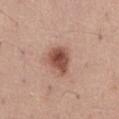Recorded during total-body skin imaging; not selected for excision or biopsy. The lesion is on the front of the torso. Cropped from a total-body skin-imaging series; the visible field is about 15 mm. Imaged with white-light lighting. The patient is a male aged 53–57.